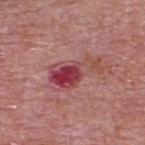| key | value |
|---|---|
| biopsy status | no biopsy performed (imaged during a skin exam) |
| illumination | white-light illumination |
| anatomic site | the upper back |
| patient | male, aged around 75 |
| size | ≈6 mm |
| imaging modality | ~15 mm tile from a whole-body skin photo |
| TBP lesion metrics | an area of roughly 12 mm², a shape eccentricity near 0.9, and two-axis asymmetry of about 0.5; a border-irregularity rating of about 6.5/10 and a within-lesion color-variation index near 9.5/10 |